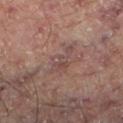Impression: Recorded during total-body skin imaging; not selected for excision or biopsy. Image and clinical context: The lesion's longest dimension is about 3.5 mm. This image is a 15 mm lesion crop taken from a total-body photograph. A male subject, aged approximately 70. The tile uses cross-polarized illumination. Located on the left lower leg.